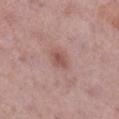{"biopsy_status": "not biopsied; imaged during a skin examination", "site": "right lower leg", "patient": {"sex": "female", "age_approx": 50}, "image": {"source": "total-body photography crop", "field_of_view_mm": 15}}